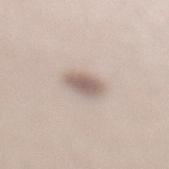Part of a total-body skin-imaging series; this lesion was reviewed on a skin check and was not flagged for biopsy. A roughly 15 mm field-of-view crop from a total-body skin photograph. On the left lower leg. Captured under white-light illumination. A female patient about 40 years old. About 3 mm across.